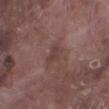This lesion was catalogued during total-body skin photography and was not selected for biopsy.
On the leg.
An algorithmic analysis of the crop reported a lesion area of about 3.5 mm², a shape eccentricity near 0.8, and a shape-asymmetry score of about 0.4 (0 = symmetric). It also reported an average lesion color of about L≈40 a*≈19 b*≈21 (CIELAB) and roughly 6 lightness units darker than nearby skin.
Cropped from a whole-body photographic skin survey; the tile spans about 15 mm.
The tile uses white-light illumination.
About 2.5 mm across.
A male subject roughly 75 years of age.